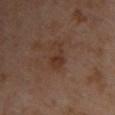Captured during whole-body skin photography for melanoma surveillance; the lesion was not biopsied. This image is a 15 mm lesion crop taken from a total-body photograph. From the upper back. The subject is a female roughly 40 years of age. About 3.5 mm across.